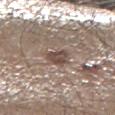A male subject about 45 years old.
Captured under white-light illumination.
The recorded lesion diameter is about 2.5 mm.
Cropped from a whole-body photographic skin survey; the tile spans about 15 mm.
On the right lower leg.